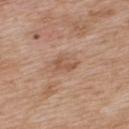Assessment:
Captured during whole-body skin photography for melanoma surveillance; the lesion was not biopsied.
Clinical summary:
A lesion tile, about 15 mm wide, cut from a 3D total-body photograph. Longest diameter approximately 2.5 mm. A female patient aged approximately 40. The tile uses white-light illumination. Located on the back. An algorithmic analysis of the crop reported a lesion area of about 3.5 mm². The analysis additionally found a classifier nevus-likeness of about 0/100.